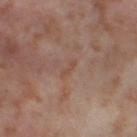Recorded during total-body skin imaging; not selected for excision or biopsy. An algorithmic analysis of the crop reported a lesion color around L≈49 a*≈19 b*≈28 in CIELAB, a lesion–skin lightness drop of about 5, and a lesion-to-skin contrast of about 5 (normalized; higher = more distinct). And it measured a border-irregularity rating of about 5/10 and a within-lesion color-variation index near 0/10. It also reported a nevus-likeness score of about 0/100 and a lesion-detection confidence of about 100/100. A 15 mm close-up tile from a total-body photography series done for melanoma screening. A female patient approximately 55 years of age. From the left thigh.